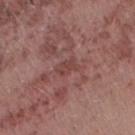workup: total-body-photography surveillance lesion; no biopsy | image-analysis metrics: a shape eccentricity near 0.85 and two-axis asymmetry of about 0.25; a mean CIELAB color near L≈43 a*≈23 b*≈22, roughly 7 lightness units darker than nearby skin, and a normalized lesion–skin contrast near 5.5 | illumination: white-light illumination | size: ≈3 mm | patient: female, aged 18 to 22 | location: the right thigh | acquisition: 15 mm crop, total-body photography.